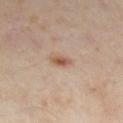workup: total-body-photography surveillance lesion; no biopsy.